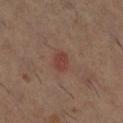Captured during whole-body skin photography for melanoma surveillance; the lesion was not biopsied.
A female patient, aged 58–62.
A 15 mm close-up tile from a total-body photography series done for melanoma screening.
About 2.5 mm across.
Captured under cross-polarized illumination.
The lesion is located on the right lower leg.